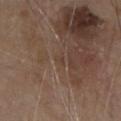Diagnosis: Histopathological examination showed an indeterminate (borderline) lesion: actinic keratosis.